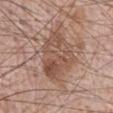The lesion was photographed on a routine skin check and not biopsied; there is no pathology result. Approximately 6.5 mm at its widest. A male patient, aged around 70. A lesion tile, about 15 mm wide, cut from a 3D total-body photograph. Imaged with white-light lighting. The lesion is on the front of the torso.